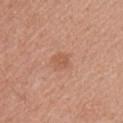Notes:
– biopsy status — catalogued during a skin exam; not biopsied
– lesion diameter — ≈2.5 mm
– location — the arm
– tile lighting — white-light illumination
– image source — 15 mm crop, total-body photography
– subject — male, about 55 years old